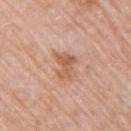{
  "biopsy_status": "not biopsied; imaged during a skin examination",
  "patient": {
    "sex": "female",
    "age_approx": 65
  },
  "site": "right upper arm",
  "image": {
    "source": "total-body photography crop",
    "field_of_view_mm": 15
  }
}